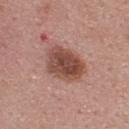Q: Was this lesion biopsied?
A: imaged on a skin check; not biopsied